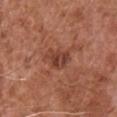  biopsy_status: not biopsied; imaged during a skin examination
  automated_metrics:
    cielab_L: 41
    cielab_a: 25
    cielab_b: 28
    vs_skin_darker_L: 9.0
    vs_skin_contrast_norm: 7.5
  site: chest
  lesion_size:
    long_diameter_mm_approx: 3.5
  patient:
    sex: male
    age_approx: 75
  image:
    source: total-body photography crop
    field_of_view_mm: 15